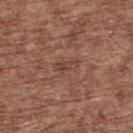follow-up: imaged on a skin check; not biopsied
patient: male, aged 73 to 77
lesion size: about 3 mm
site: the upper back
imaging modality: ~15 mm crop, total-body skin-cancer survey
tile lighting: white-light illumination